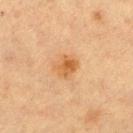Q: Was this lesion biopsied?
A: no biopsy performed (imaged during a skin exam)
Q: What lighting was used for the tile?
A: cross-polarized illumination
Q: What kind of image is this?
A: 15 mm crop, total-body photography
Q: What are the patient's age and sex?
A: female, roughly 55 years of age
Q: What did automated image analysis measure?
A: a mean CIELAB color near L≈50 a*≈20 b*≈37, about 8 CIELAB-L* units darker than the surrounding skin, and a normalized lesion–skin contrast near 7.5; a border-irregularity index near 2/10, a within-lesion color-variation index near 4.5/10, and a peripheral color-asymmetry measure near 2; a nevus-likeness score of about 75/100
Q: Where on the body is the lesion?
A: the leg